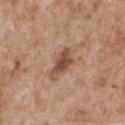patient:
  sex: male
  age_approx: 65
lighting: white-light
image:
  source: total-body photography crop
  field_of_view_mm: 15
site: chest
lesion_size:
  long_diameter_mm_approx: 4.0